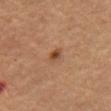This lesion was catalogued during total-body skin photography and was not selected for biopsy. Cropped from a whole-body photographic skin survey; the tile spans about 15 mm. A male subject approximately 65 years of age. The lesion is located on the mid back.